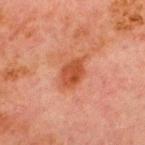{
  "biopsy_status": "not biopsied; imaged during a skin examination",
  "image": {
    "source": "total-body photography crop",
    "field_of_view_mm": 15
  },
  "patient": {
    "sex": "male",
    "age_approx": 70
  },
  "lighting": "cross-polarized",
  "lesion_size": {
    "long_diameter_mm_approx": 4.0
  },
  "site": "chest",
  "automated_metrics": {
    "area_mm2_approx": 7.0,
    "eccentricity": 0.75,
    "shape_asymmetry": 0.25,
    "cielab_L": 42,
    "cielab_a": 27,
    "cielab_b": 33,
    "vs_skin_darker_L": 9.0,
    "vs_skin_contrast_norm": 8.5,
    "color_variation_0_10": 2.5,
    "nevus_likeness_0_100": 65,
    "lesion_detection_confidence_0_100": 100
  }
}